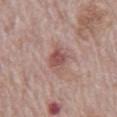notes = no biopsy performed (imaged during a skin exam); body site = the abdomen; patient = male, approximately 70 years of age; imaging modality = total-body-photography crop, ~15 mm field of view; lesion size = about 3.5 mm.